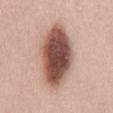| key | value |
|---|---|
| notes | total-body-photography surveillance lesion; no biopsy |
| subject | male, aged 43–47 |
| image-analysis metrics | a lesion area of about 30 mm² and an outline eccentricity of about 0.85 (0 = round, 1 = elongated); an average lesion color of about L≈52 a*≈21 b*≈24 (CIELAB); a peripheral color-asymmetry measure near 2.5 |
| image source | ~15 mm crop, total-body skin-cancer survey |
| tile lighting | white-light |
| body site | the abdomen |
| diameter | about 9 mm |